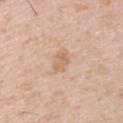follow-up = total-body-photography surveillance lesion; no biopsy | lighting = white-light | image source = ~15 mm tile from a whole-body skin photo | patient = male, aged approximately 50 | site = the right upper arm | lesion size = about 3 mm.